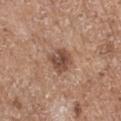The lesion was tiled from a total-body skin photograph and was not biopsied.
An algorithmic analysis of the crop reported an area of roughly 7 mm², an outline eccentricity of about 0.5 (0 = round, 1 = elongated), and a shape-asymmetry score of about 0.2 (0 = symmetric). And it measured roughly 12 lightness units darker than nearby skin.
About 3 mm across.
A male patient aged 68–72.
Located on the left upper arm.
A 15 mm close-up tile from a total-body photography series done for melanoma screening.
Imaged with white-light lighting.